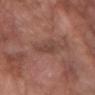{"biopsy_status": "not biopsied; imaged during a skin examination", "patient": {"sex": "female", "age_approx": 70}, "image": {"source": "total-body photography crop", "field_of_view_mm": 15}, "site": "left forearm"}